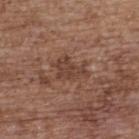Impression:
Captured during whole-body skin photography for melanoma surveillance; the lesion was not biopsied.
Clinical summary:
Located on the upper back. A lesion tile, about 15 mm wide, cut from a 3D total-body photograph. A female patient aged 63–67.